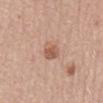Captured during whole-body skin photography for melanoma surveillance; the lesion was not biopsied.